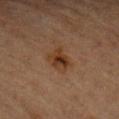Case summary:
– workup: catalogued during a skin exam; not biopsied
– image source: ~15 mm tile from a whole-body skin photo
– body site: the arm
– patient: female, aged approximately 65